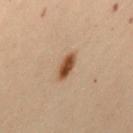Clinical impression:
The lesion was tiled from a total-body skin photograph and was not biopsied.
Clinical summary:
A female subject, in their 50s. A 15 mm close-up tile from a total-body photography series done for melanoma screening. The tile uses cross-polarized illumination. Longest diameter approximately 3 mm. Located on the mid back.